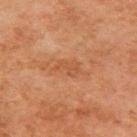Clinical impression:
This lesion was catalogued during total-body skin photography and was not selected for biopsy.